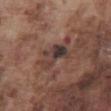Impression: The lesion was photographed on a routine skin check and not biopsied; there is no pathology result.